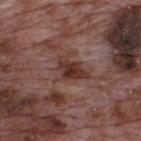| feature | finding |
|---|---|
| follow-up | catalogued during a skin exam; not biopsied |
| site | the upper back |
| illumination | white-light |
| image source | ~15 mm crop, total-body skin-cancer survey |
| subject | male, roughly 70 years of age |
| automated lesion analysis | border irregularity of about 4 on a 0–10 scale, a within-lesion color-variation index near 3.5/10, and peripheral color asymmetry of about 1; a classifier nevus-likeness of about 45/100 |
| size | ~3.5 mm (longest diameter) |